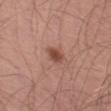No biopsy was performed on this lesion — it was imaged during a full skin examination and was not determined to be concerning.
From the left thigh.
About 2.5 mm across.
Imaged with white-light lighting.
A male patient, aged 68 to 72.
The lesion-visualizer software estimated a lesion area of about 3.5 mm². It also reported a mean CIELAB color near L≈47 a*≈24 b*≈27, roughly 11 lightness units darker than nearby skin, and a normalized border contrast of about 8.5. The analysis additionally found a border-irregularity index near 2/10 and a peripheral color-asymmetry measure near 0.5.
A 15 mm crop from a total-body photograph taken for skin-cancer surveillance.